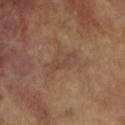| key | value |
|---|---|
| workup | catalogued during a skin exam; not biopsied |
| illumination | cross-polarized |
| body site | the right forearm |
| patient | female, aged around 75 |
| imaging modality | ~15 mm tile from a whole-body skin photo |
| lesion size | ~3.5 mm (longest diameter) |